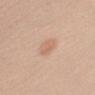The lesion was tiled from a total-body skin photograph and was not biopsied.
A 15 mm crop from a total-body photograph taken for skin-cancer surveillance.
Automated image analysis of the tile measured a color-variation rating of about 1.5/10 and peripheral color asymmetry of about 0.5.
Located on the left upper arm.
A male subject, aged approximately 40.